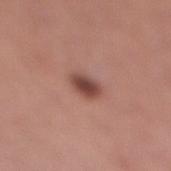Assessment:
Recorded during total-body skin imaging; not selected for excision or biopsy.
Clinical summary:
A female subject, about 60 years old. The lesion is located on the leg. A region of skin cropped from a whole-body photographic capture, roughly 15 mm wide.